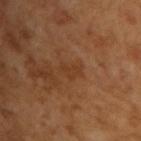Recorded during total-body skin imaging; not selected for excision or biopsy. The patient is a male approximately 65 years of age. The recorded lesion diameter is about 2.5 mm. Cropped from a total-body skin-imaging series; the visible field is about 15 mm. The lesion-visualizer software estimated a shape eccentricity near 0.8. The analysis additionally found an average lesion color of about L≈36 a*≈21 b*≈32 (CIELAB), a lesion–skin lightness drop of about 5, and a normalized border contrast of about 5. And it measured a border-irregularity rating of about 4.5/10 and a color-variation rating of about 0/10.